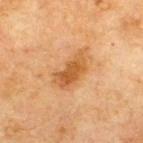biopsy_status: not biopsied; imaged during a skin examination
automated_metrics:
  area_mm2_approx: 9.5
  eccentricity: 0.85
  shape_asymmetry: 0.3
  nevus_likeness_0_100: 50
image:
  source: total-body photography crop
  field_of_view_mm: 15
lesion_size:
  long_diameter_mm_approx: 4.5
patient:
  sex: male
  age_approx: 70
site: upper back